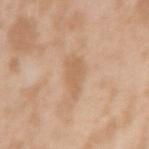{"biopsy_status": "not biopsied; imaged during a skin examination", "lighting": "white-light", "image": {"source": "total-body photography crop", "field_of_view_mm": 15}, "patient": {"sex": "male", "age_approx": 45}, "site": "right upper arm", "automated_metrics": {"area_mm2_approx": 6.5, "shape_asymmetry": 0.35, "color_variation_0_10": 1.5, "nevus_likeness_0_100": 0, "lesion_detection_confidence_0_100": 100}}